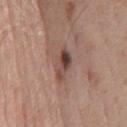workup = total-body-photography surveillance lesion; no biopsy
patient = male, aged 58 to 62
size = about 4.5 mm
illumination = white-light
site = the chest
acquisition = total-body-photography crop, ~15 mm field of view